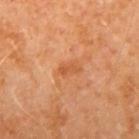biopsy status = imaged on a skin check; not biopsied
size = about 3 mm
lighting = cross-polarized
location = the right upper arm
imaging modality = total-body-photography crop, ~15 mm field of view
patient = male, approximately 60 years of age
automated metrics = an eccentricity of roughly 0.8 and two-axis asymmetry of about 0.2; border irregularity of about 2 on a 0–10 scale and peripheral color asymmetry of about 0.5